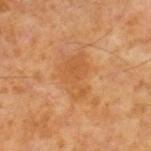Q: Was a biopsy performed?
A: no biopsy performed (imaged during a skin exam)
Q: Illumination type?
A: cross-polarized
Q: What is the anatomic site?
A: the right lower leg
Q: Lesion size?
A: ~5 mm (longest diameter)
Q: What are the patient's age and sex?
A: male, in their 60s
Q: How was this image acquired?
A: ~15 mm crop, total-body skin-cancer survey